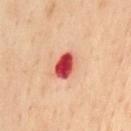About 3.5 mm across.
The tile uses cross-polarized illumination.
This image is a 15 mm lesion crop taken from a total-body photograph.
The patient is a male roughly 55 years of age.
The lesion is on the chest.